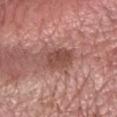Impression: The lesion was photographed on a routine skin check and not biopsied; there is no pathology result. Clinical summary: A male patient, aged around 60. Located on the arm. Automated tile analysis of the lesion measured an area of roughly 8.5 mm², an outline eccentricity of about 0.75 (0 = round, 1 = elongated), and a shape-asymmetry score of about 0.15 (0 = symmetric). The software also gave a lesion–skin lightness drop of about 11. The software also gave border irregularity of about 2 on a 0–10 scale, a color-variation rating of about 3/10, and a peripheral color-asymmetry measure near 1. And it measured an automated nevus-likeness rating near 35 out of 100 and a detector confidence of about 100 out of 100 that the crop contains a lesion. The tile uses white-light illumination. This image is a 15 mm lesion crop taken from a total-body photograph.